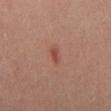- notes — total-body-photography surveillance lesion; no biopsy
- site — the mid back
- subject — male, aged approximately 45
- size — ≈3 mm
- image — ~15 mm crop, total-body skin-cancer survey